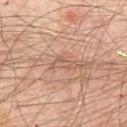follow-up=no biopsy performed (imaged during a skin exam).